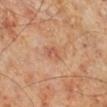The lesion was photographed on a routine skin check and not biopsied; there is no pathology result.